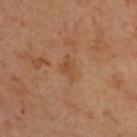follow-up=no biopsy performed (imaged during a skin exam) | body site=the upper back | subject=male, aged 43–47 | lesion diameter=about 2.5 mm | acquisition=~15 mm tile from a whole-body skin photo.